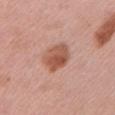biopsy_status: not biopsied; imaged during a skin examination
lesion_size:
  long_diameter_mm_approx: 3.5
lighting: white-light
patient:
  sex: female
  age_approx: 45
site: arm
automated_metrics:
  cielab_L: 54
  cielab_a: 25
  cielab_b: 30
  vs_skin_darker_L: 12.0
  vs_skin_contrast_norm: 9.0
  border_irregularity_0_10: 1.5
  nevus_likeness_0_100: 90
  lesion_detection_confidence_0_100: 100
image:
  source: total-body photography crop
  field_of_view_mm: 15